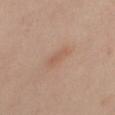Part of a total-body skin-imaging series; this lesion was reviewed on a skin check and was not flagged for biopsy.
A 15 mm close-up tile from a total-body photography series done for melanoma screening.
The patient is a female aged 38 to 42.
Measured at roughly 3.5 mm in maximum diameter.
This is a cross-polarized tile.
The lesion is located on the left leg.